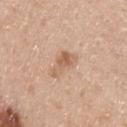Clinical impression: This lesion was catalogued during total-body skin photography and was not selected for biopsy. Acquisition and patient details: The lesion's longest dimension is about 4 mm. The tile uses white-light illumination. A 15 mm crop from a total-body photograph taken for skin-cancer surveillance. The subject is a male aged around 40. The lesion-visualizer software estimated a border-irregularity index near 5/10. The analysis additionally found a classifier nevus-likeness of about 20/100 and lesion-presence confidence of about 100/100. From the upper back.